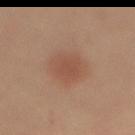Clinical impression:
The lesion was photographed on a routine skin check and not biopsied; there is no pathology result.
Context:
The lesion is located on the mid back. A female patient aged approximately 45. Cropped from a whole-body photographic skin survey; the tile spans about 15 mm. The tile uses cross-polarized illumination. The recorded lesion diameter is about 4.5 mm.